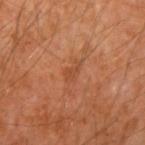Assessment:
Recorded during total-body skin imaging; not selected for excision or biopsy.
Image and clinical context:
A male subject, approximately 60 years of age. This image is a 15 mm lesion crop taken from a total-body photograph. Located on the right upper arm.